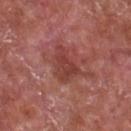<case>
<biopsy_status>not biopsied; imaged during a skin examination</biopsy_status>
<lesion_size>
  <long_diameter_mm_approx>4.5</long_diameter_mm_approx>
</lesion_size>
<site>chest</site>
<image>
  <source>total-body photography crop</source>
  <field_of_view_mm>15</field_of_view_mm>
</image>
<lighting>white-light</lighting>
<patient>
  <sex>male</sex>
  <age_approx>65</age_approx>
</patient>
</case>